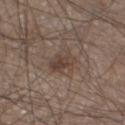The lesion was photographed on a routine skin check and not biopsied; there is no pathology result.